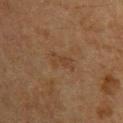– workup: no biopsy performed (imaged during a skin exam)
– illumination: cross-polarized illumination
– patient: male, roughly 60 years of age
– image source: total-body-photography crop, ~15 mm field of view
– lesion size: about 3.5 mm